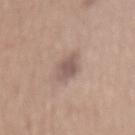notes: total-body-photography surveillance lesion; no biopsy
lighting: white-light
location: the lower back
imaging modality: 15 mm crop, total-body photography
diameter: ~3 mm (longest diameter)
patient: male, about 65 years old